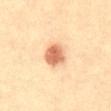Recorded during total-body skin imaging; not selected for excision or biopsy. The lesion is on the abdomen. Captured under cross-polarized illumination. A roughly 15 mm field-of-view crop from a total-body skin photograph. A male patient roughly 35 years of age. An algorithmic analysis of the crop reported a lesion area of about 7 mm², an eccentricity of roughly 0.4, and a shape-asymmetry score of about 0.15 (0 = symmetric). It also reported a border-irregularity index near 1/10, internal color variation of about 3 on a 0–10 scale, and a peripheral color-asymmetry measure near 1. It also reported a lesion-detection confidence of about 100/100.